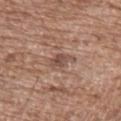biopsy_status: not biopsied; imaged during a skin examination